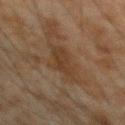Notes:
– notes: total-body-photography surveillance lesion; no biopsy
– lighting: cross-polarized
– image-analysis metrics: a border-irregularity index near 4.5/10, internal color variation of about 2 on a 0–10 scale, and radial color variation of about 0.5; lesion-presence confidence of about 100/100
– patient: male, approximately 45 years of age
– image source: ~15 mm crop, total-body skin-cancer survey
– size: ≈4.5 mm
– body site: the left forearm